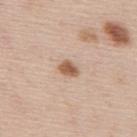follow-up: imaged on a skin check; not biopsied
subject: male, about 45 years old
body site: the back
automated lesion analysis: a footprint of about 4 mm² and an outline eccentricity of about 0.65 (0 = round, 1 = elongated); an average lesion color of about L≈58 a*≈19 b*≈31 (CIELAB), about 13 CIELAB-L* units darker than the surrounding skin, and a normalized border contrast of about 9; a border-irregularity index near 2/10 and a within-lesion color-variation index near 2.5/10
tile lighting: white-light illumination
lesion diameter: ≈2.5 mm
image source: 15 mm crop, total-body photography